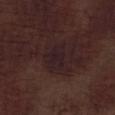Impression:
The lesion was photographed on a routine skin check and not biopsied; there is no pathology result.
Context:
Automated tile analysis of the lesion measured a lesion color around L≈19 a*≈14 b*≈11 in CIELAB and a normalized border contrast of about 7.5. A 15 mm close-up extracted from a 3D total-body photography capture. The lesion's longest dimension is about 3 mm. A male subject aged 68–72. The lesion is located on the left lower leg.